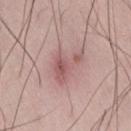Part of a total-body skin-imaging series; this lesion was reviewed on a skin check and was not flagged for biopsy.
A close-up tile cropped from a whole-body skin photograph, about 15 mm across.
The lesion is on the back.
The patient is a male aged 48–52.
This is a white-light tile.